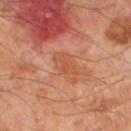Assessment: The lesion was tiled from a total-body skin photograph and was not biopsied. Background: The recorded lesion diameter is about 4 mm. A male patient, aged around 70. A lesion tile, about 15 mm wide, cut from a 3D total-body photograph. Located on the left lower leg.